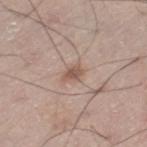tile lighting — white-light | patient — male, about 70 years old | TBP lesion metrics — an eccentricity of roughly 0.75 and a shape-asymmetry score of about 0.25 (0 = symmetric); a lesion-to-skin contrast of about 7 (normalized; higher = more distinct); an automated nevus-likeness rating near 65 out of 100 | location — the right leg | image source — ~15 mm crop, total-body skin-cancer survey | lesion size — ≈2.5 mm.